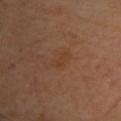workup: total-body-photography surveillance lesion; no biopsy
lesion diameter: ≈2.5 mm
image source: 15 mm crop, total-body photography
subject: female, aged approximately 60
body site: the upper back
lighting: cross-polarized